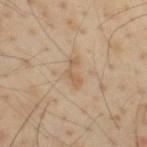| key | value |
|---|---|
| workup | no biopsy performed (imaged during a skin exam) |
| patient | male, aged approximately 55 |
| location | the upper back |
| imaging modality | ~15 mm crop, total-body skin-cancer survey |